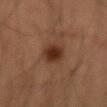{
  "biopsy_status": "not biopsied; imaged during a skin examination",
  "site": "right forearm",
  "lesion_size": {
    "long_diameter_mm_approx": 3.0
  },
  "patient": {
    "sex": "male",
    "age_approx": 55
  },
  "lighting": "cross-polarized",
  "image": {
    "source": "total-body photography crop",
    "field_of_view_mm": 15
  }
}